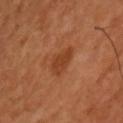Part of a total-body skin-imaging series; this lesion was reviewed on a skin check and was not flagged for biopsy. A close-up tile cropped from a whole-body skin photograph, about 15 mm across. Located on the arm. A male subject, roughly 50 years of age.